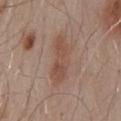Clinical impression:
The lesion was tiled from a total-body skin photograph and was not biopsied.